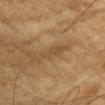notes: catalogued during a skin exam; not biopsied | site: the chest | image: total-body-photography crop, ~15 mm field of view | lighting: cross-polarized illumination | patient: male, roughly 60 years of age | diameter: ~3 mm (longest diameter).